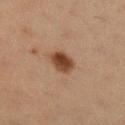workup: catalogued during a skin exam; not biopsied | subject: female, aged 38–42 | tile lighting: cross-polarized | automated lesion analysis: a footprint of about 6 mm²; a lesion-detection confidence of about 100/100 | diameter: ≈3.5 mm | body site: the right lower leg | acquisition: ~15 mm tile from a whole-body skin photo.